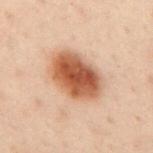notes: catalogued during a skin exam; not biopsied | size: ~6 mm (longest diameter) | tile lighting: cross-polarized | automated lesion analysis: a lesion area of about 21 mm², a shape eccentricity near 0.75, and two-axis asymmetry of about 0.15; a mean CIELAB color near L≈46 a*≈21 b*≈29, roughly 15 lightness units darker than nearby skin, and a normalized lesion–skin contrast near 11; a border-irregularity index near 1.5/10; an automated nevus-likeness rating near 100 out of 100 | subject: male, aged approximately 50 | site: the mid back | imaging modality: 15 mm crop, total-body photography.